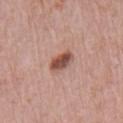notes — total-body-photography surveillance lesion; no biopsy.